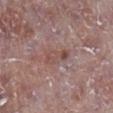Recorded during total-body skin imaging; not selected for excision or biopsy. The lesion-visualizer software estimated a lesion area of about 6 mm² and two-axis asymmetry of about 0.25. And it measured a classifier nevus-likeness of about 0/100 and a detector confidence of about 100 out of 100 that the crop contains a lesion. Cropped from a total-body skin-imaging series; the visible field is about 15 mm. On the leg. Longest diameter approximately 4 mm. A male subject in their mid-70s.